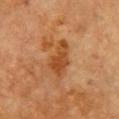Q: Was a biopsy performed?
A: total-body-photography surveillance lesion; no biopsy
Q: Who is the patient?
A: male, about 85 years old or older
Q: Lesion location?
A: the chest
Q: Illumination type?
A: cross-polarized
Q: Lesion size?
A: ~5 mm (longest diameter)
Q: What kind of image is this?
A: ~15 mm tile from a whole-body skin photo